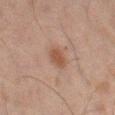notes = imaged on a skin check; not biopsied | subject = male, approximately 45 years of age | site = the left thigh | imaging modality = total-body-photography crop, ~15 mm field of view | automated metrics = a footprint of about 4.5 mm², an eccentricity of roughly 0.75, and two-axis asymmetry of about 0.15; a border-irregularity rating of about 1.5/10 and radial color variation of about 0.5 | lesion diameter = ~3 mm (longest diameter).